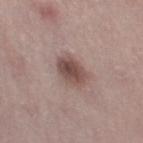<case>
<biopsy_status>not biopsied; imaged during a skin examination</biopsy_status>
<patient>
  <sex>female</sex>
  <age_approx>45</age_approx>
</patient>
<site>left thigh</site>
<image>
  <source>total-body photography crop</source>
  <field_of_view_mm>15</field_of_view_mm>
</image>
<automated_metrics>
  <border_irregularity_0_10>1.5</border_irregularity_0_10>
  <color_variation_0_10>5.0</color_variation_0_10>
  <peripheral_color_asymmetry>1.5</peripheral_color_asymmetry>
</automated_metrics>
</case>